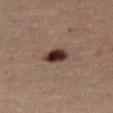The lesion was photographed on a routine skin check and not biopsied; there is no pathology result. The lesion's longest dimension is about 3 mm. A 15 mm close-up extracted from a 3D total-body photography capture. The lesion is located on the right thigh. The patient is a female roughly 45 years of age. This is a cross-polarized tile. An algorithmic analysis of the crop reported a lesion color around L≈32 a*≈18 b*≈23 in CIELAB, roughly 18 lightness units darker than nearby skin, and a normalized lesion–skin contrast near 15. The software also gave border irregularity of about 2 on a 0–10 scale, internal color variation of about 5 on a 0–10 scale, and radial color variation of about 1.5. And it measured a classifier nevus-likeness of about 100/100.